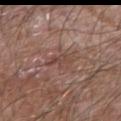| feature | finding |
|---|---|
| biopsy status | no biopsy performed (imaged during a skin exam) |